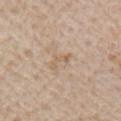| feature | finding |
|---|---|
| location | the right upper arm |
| lesion diameter | about 3 mm |
| patient | male, approximately 75 years of age |
| imaging modality | ~15 mm tile from a whole-body skin photo |